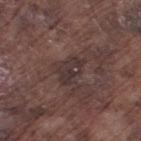This lesion was catalogued during total-body skin photography and was not selected for biopsy. About 3.5 mm across. This image is a 15 mm lesion crop taken from a total-body photograph. The lesion is on the right thigh. The subject is a male in their mid-70s.